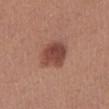| key | value |
|---|---|
| follow-up | no biopsy performed (imaged during a skin exam) |
| imaging modality | 15 mm crop, total-body photography |
| anatomic site | the right lower leg |
| patient | female, aged around 40 |